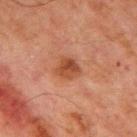<lesion>
<biopsy_status>not biopsied; imaged during a skin examination</biopsy_status>
<patient>
  <sex>male</sex>
  <age_approx>65</age_approx>
</patient>
<site>chest</site>
<automated_metrics>
  <eccentricity>0.35</eccentricity>
  <cielab_L>38</cielab_L>
  <cielab_a>23</cielab_a>
  <cielab_b>29</cielab_b>
  <vs_skin_darker_L>9.0</vs_skin_darker_L>
  <vs_skin_contrast_norm>8.0</vs_skin_contrast_norm>
  <nevus_likeness_0_100>35</nevus_likeness_0_100>
  <lesion_detection_confidence_0_100>100</lesion_detection_confidence_0_100>
</automated_metrics>
<image>
  <source>total-body photography crop</source>
  <field_of_view_mm>15</field_of_view_mm>
</image>
<lesion_size>
  <long_diameter_mm_approx>3.0</long_diameter_mm_approx>
</lesion_size>
<lighting>cross-polarized</lighting>
</lesion>